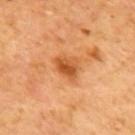biopsy status=catalogued during a skin exam; not biopsied.